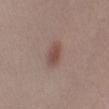diameter: ~3.5 mm (longest diameter) | image-analysis metrics: an outline eccentricity of about 0.85 (0 = round, 1 = elongated) and a shape-asymmetry score of about 0.15 (0 = symmetric); a border-irregularity index near 1.5/10, a within-lesion color-variation index near 2/10, and peripheral color asymmetry of about 0.5 | image source: 15 mm crop, total-body photography | patient: female, aged around 20 | body site: the left lower leg.